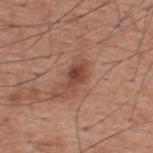No biopsy was performed on this lesion — it was imaged during a full skin examination and was not determined to be concerning.
Approximately 3.5 mm at its widest.
Automated image analysis of the tile measured roughly 11 lightness units darker than nearby skin and a normalized lesion–skin contrast near 8. The analysis additionally found a classifier nevus-likeness of about 35/100 and a detector confidence of about 100 out of 100 that the crop contains a lesion.
The subject is a male aged 58 to 62.
A 15 mm close-up extracted from a 3D total-body photography capture.
From the back.